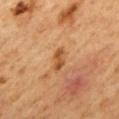| field | value |
|---|---|
| notes | catalogued during a skin exam; not biopsied |
| subject | male, aged 58–62 |
| site | the back |
| image source | ~15 mm tile from a whole-body skin photo |
| illumination | cross-polarized |